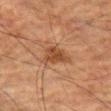Assessment:
No biopsy was performed on this lesion — it was imaged during a full skin examination and was not determined to be concerning.